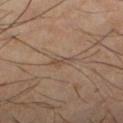Q: Is there a histopathology result?
A: no biopsy performed (imaged during a skin exam)
Q: Where on the body is the lesion?
A: the left lower leg
Q: Who is the patient?
A: male, aged around 40
Q: What is the imaging modality?
A: 15 mm crop, total-body photography
Q: How was the tile lit?
A: cross-polarized
Q: Lesion size?
A: ~3 mm (longest diameter)
Q: What did automated image analysis measure?
A: a border-irregularity index near 5.5/10, internal color variation of about 0 on a 0–10 scale, and a peripheral color-asymmetry measure near 0